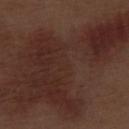{"biopsy_status": "not biopsied; imaged during a skin examination", "site": "right thigh", "automated_metrics": {"area_mm2_approx": 95.0, "shape_asymmetry": 0.55, "color_variation_0_10": 4.0, "peripheral_color_asymmetry": 1.5}, "lighting": "white-light", "patient": {"sex": "male", "age_approx": 70}, "lesion_size": {"long_diameter_mm_approx": 18.5}, "image": {"source": "total-body photography crop", "field_of_view_mm": 15}}